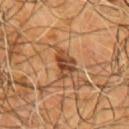Clinical impression: The lesion was tiled from a total-body skin photograph and was not biopsied. Clinical summary: A male patient approximately 55 years of age. The lesion is located on the chest. The tile uses cross-polarized illumination. A lesion tile, about 15 mm wide, cut from a 3D total-body photograph.